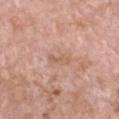- notes — catalogued during a skin exam; not biopsied
- size — ~2.5 mm (longest diameter)
- patient — female, in their mid-70s
- anatomic site — the chest
- acquisition — ~15 mm tile from a whole-body skin photo